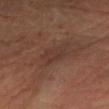Case summary:
– biopsy status — no biopsy performed (imaged during a skin exam)
– subject — male, roughly 55 years of age
– size — ~3 mm (longest diameter)
– acquisition — 15 mm crop, total-body photography
– automated lesion analysis — a lesion color around L≈33 a*≈18 b*≈23 in CIELAB and about 5 CIELAB-L* units darker than the surrounding skin; a detector confidence of about 80 out of 100 that the crop contains a lesion
– body site — the left forearm
– lighting — cross-polarized